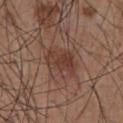The lesion was photographed on a routine skin check and not biopsied; there is no pathology result.
A male patient, aged approximately 55.
Captured under white-light illumination.
On the abdomen.
A 15 mm close-up extracted from a 3D total-body photography capture.
The total-body-photography lesion software estimated a nevus-likeness score of about 35/100.
The recorded lesion diameter is about 4 mm.